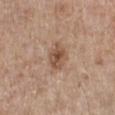Assessment:
This lesion was catalogued during total-body skin photography and was not selected for biopsy.
Acquisition and patient details:
On the right lower leg. Cropped from a total-body skin-imaging series; the visible field is about 15 mm. The lesion's longest dimension is about 3.5 mm. The patient is a female aged 58 to 62.